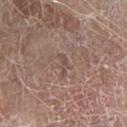Imaged during a routine full-body skin examination; the lesion was not biopsied and no histopathology is available.
Measured at roughly 2.5 mm in maximum diameter.
A 15 mm crop from a total-body photograph taken for skin-cancer surveillance.
This is a white-light tile.
An algorithmic analysis of the crop reported a border-irregularity rating of about 4.5/10, internal color variation of about 0 on a 0–10 scale, and peripheral color asymmetry of about 0.
A male patient about 80 years old.
The lesion is on the right forearm.